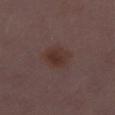<tbp_lesion>
  <biopsy_status>not biopsied; imaged during a skin examination</biopsy_status>
  <lighting>white-light</lighting>
  <lesion_size>
    <long_diameter_mm_approx>3.5</long_diameter_mm_approx>
  </lesion_size>
  <image>
    <source>total-body photography crop</source>
    <field_of_view_mm>15</field_of_view_mm>
  </image>
  <patient>
    <sex>female</sex>
    <age_approx>30</age_approx>
  </patient>
  <site>leg</site>
</tbp_lesion>